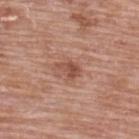This lesion was catalogued during total-body skin photography and was not selected for biopsy. Imaged with white-light lighting. A 15 mm close-up tile from a total-body photography series done for melanoma screening. Automated image analysis of the tile measured a footprint of about 4 mm², an outline eccentricity of about 0.75 (0 = round, 1 = elongated), and a symmetry-axis asymmetry near 0.35. The analysis additionally found a mean CIELAB color near L≈50 a*≈24 b*≈29, about 10 CIELAB-L* units darker than the surrounding skin, and a normalized lesion–skin contrast near 7. The analysis additionally found a border-irregularity rating of about 3.5/10, a color-variation rating of about 2.5/10, and peripheral color asymmetry of about 1. It also reported a nevus-likeness score of about 20/100 and a detector confidence of about 100 out of 100 that the crop contains a lesion. A female subject, in their 60s. The lesion is on the upper back.